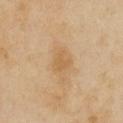Clinical summary: Cropped from a total-body skin-imaging series; the visible field is about 15 mm. A female subject aged approximately 40. Located on the chest. About 2.5 mm across.